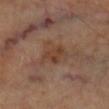follow-up = no biopsy performed (imaged during a skin exam)
TBP lesion metrics = a nevus-likeness score of about 0/100
lighting = cross-polarized illumination
acquisition = 15 mm crop, total-body photography
diameter = about 2.5 mm
site = the left leg
patient = female, aged 58–62